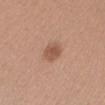Recorded during total-body skin imaging; not selected for excision or biopsy.
A 15 mm crop from a total-body photograph taken for skin-cancer surveillance.
An algorithmic analysis of the crop reported a footprint of about 4.5 mm² and two-axis asymmetry of about 0.2. The software also gave an average lesion color of about L≈53 a*≈21 b*≈29 (CIELAB), a lesion–skin lightness drop of about 10, and a normalized border contrast of about 7.
The subject is a female aged 43 to 47.
Located on the head or neck.